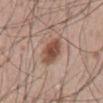Captured during whole-body skin photography for melanoma surveillance; the lesion was not biopsied.
On the abdomen.
Automated image analysis of the tile measured a mean CIELAB color near L≈50 a*≈20 b*≈28, about 12 CIELAB-L* units darker than the surrounding skin, and a normalized lesion–skin contrast near 9. It also reported an automated nevus-likeness rating near 100 out of 100 and a lesion-detection confidence of about 100/100.
The lesion's longest dimension is about 3.5 mm.
Imaged with white-light lighting.
A close-up tile cropped from a whole-body skin photograph, about 15 mm across.
A male patient, about 55 years old.